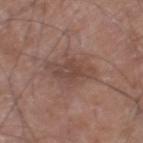Q: Is there a histopathology result?
A: imaged on a skin check; not biopsied
Q: Patient demographics?
A: male, in their 60s
Q: How large is the lesion?
A: about 4.5 mm
Q: Automated lesion metrics?
A: a footprint of about 12 mm², a shape eccentricity near 0.75, and a shape-asymmetry score of about 0.3 (0 = symmetric); a lesion color around L≈45 a*≈18 b*≈23 in CIELAB, a lesion–skin lightness drop of about 8, and a normalized lesion–skin contrast near 6
Q: What is the anatomic site?
A: the leg
Q: What is the imaging modality?
A: 15 mm crop, total-body photography